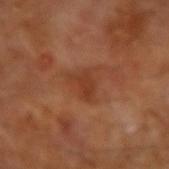Imaged during a routine full-body skin examination; the lesion was not biopsied and no histopathology is available. About 3 mm across. The tile uses cross-polarized illumination. A close-up tile cropped from a whole-body skin photograph, about 15 mm across. The patient is a male roughly 65 years of age.